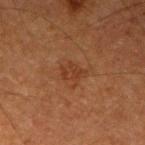lighting: cross-polarized
patient:
  sex: male
  age_approx: 75
site: arm
automated_metrics:
  area_mm2_approx: 5.0
  eccentricity: 0.6
  shape_asymmetry: 0.35
  cielab_L: 30
  cielab_a: 20
  cielab_b: 28
  vs_skin_darker_L: 5.0
  vs_skin_contrast_norm: 5.5
  border_irregularity_0_10: 4.5
  color_variation_0_10: 1.5
  peripheral_color_asymmetry: 0.5
  lesion_detection_confidence_0_100: 100
lesion_size:
  long_diameter_mm_approx: 2.5
image:
  source: total-body photography crop
  field_of_view_mm: 15